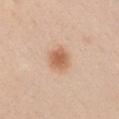Notes:
– notes: total-body-photography surveillance lesion; no biopsy
– image source: 15 mm crop, total-body photography
– site: the left upper arm
– patient: male, in their mid-30s